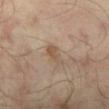Imaged during a routine full-body skin examination; the lesion was not biopsied and no histopathology is available. The lesion is located on the left lower leg. A 15 mm close-up tile from a total-body photography series done for melanoma screening. A male subject, approximately 65 years of age. Imaged with cross-polarized lighting. Longest diameter approximately 3 mm.